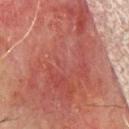Q: What did automated image analysis measure?
A: a shape eccentricity near 0.85; a normalized border contrast of about 6.5; a border-irregularity index near 8/10, a within-lesion color-variation index near 5.5/10, and radial color variation of about 1.5; lesion-presence confidence of about 85/100
Q: What is the anatomic site?
A: the chest
Q: What is the imaging modality?
A: ~15 mm tile from a whole-body skin photo
Q: What are the patient's age and sex?
A: male, about 70 years old
Q: Illumination type?
A: cross-polarized